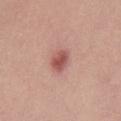Q: Is there a histopathology result?
A: catalogued during a skin exam; not biopsied
Q: What kind of image is this?
A: 15 mm crop, total-body photography
Q: Who is the patient?
A: male, roughly 30 years of age
Q: What lighting was used for the tile?
A: white-light
Q: What is the lesion's diameter?
A: about 3 mm